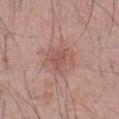biopsy status = catalogued during a skin exam; not biopsied | body site = the abdomen | automated metrics = a footprint of about 13 mm², a shape eccentricity near 0.4, and a symmetry-axis asymmetry near 0.15; a border-irregularity index near 2/10, a within-lesion color-variation index near 3.5/10, and peripheral color asymmetry of about 1 | tile lighting = white-light illumination | subject = male, aged approximately 70 | acquisition = total-body-photography crop, ~15 mm field of view.